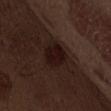<lesion>
<biopsy_status>not biopsied; imaged during a skin examination</biopsy_status>
<image>
  <source>total-body photography crop</source>
  <field_of_view_mm>15</field_of_view_mm>
</image>
<lighting>white-light</lighting>
<lesion_size>
  <long_diameter_mm_approx>5.0</long_diameter_mm_approx>
</lesion_size>
<patient>
  <sex>male</sex>
  <age_approx>70</age_approx>
</patient>
<automated_metrics>
  <area_mm2_approx>12.0</area_mm2_approx>
  <shape_asymmetry>0.2</shape_asymmetry>
</automated_metrics>
<site>abdomen</site>
</lesion>